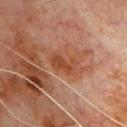No biopsy was performed on this lesion — it was imaged during a full skin examination and was not determined to be concerning. A male patient in their 80s. A 15 mm close-up extracted from a 3D total-body photography capture. The lesion is located on the chest. An algorithmic analysis of the crop reported a color-variation rating of about 2.5/10 and a peripheral color-asymmetry measure near 1. Measured at roughly 3.5 mm in maximum diameter.